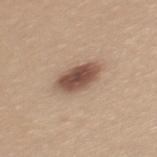Q: Was this lesion biopsied?
A: no biopsy performed (imaged during a skin exam)
Q: Lesion location?
A: the back
Q: What lighting was used for the tile?
A: white-light
Q: Automated lesion metrics?
A: a mean CIELAB color near L≈50 a*≈19 b*≈26, a lesion–skin lightness drop of about 16, and a normalized border contrast of about 11; a border-irregularity index near 2/10, a within-lesion color-variation index near 4.5/10, and radial color variation of about 1.5; a nevus-likeness score of about 95/100 and a lesion-detection confidence of about 100/100
Q: What is the imaging modality?
A: total-body-photography crop, ~15 mm field of view
Q: Lesion size?
A: about 4.5 mm
Q: What are the patient's age and sex?
A: female, in their mid- to late 20s